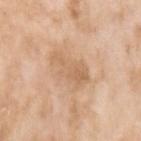notes: no biopsy performed (imaged during a skin exam) | size: about 4.5 mm | site: the left upper arm | acquisition: ~15 mm crop, total-body skin-cancer survey | subject: female, roughly 75 years of age | illumination: white-light.